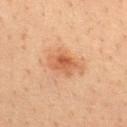The tile uses cross-polarized illumination. A male patient in their mid-30s. Located on the upper back. The recorded lesion diameter is about 5 mm. Cropped from a whole-body photographic skin survey; the tile spans about 15 mm. Automated image analysis of the tile measured an average lesion color of about L≈56 a*≈24 b*≈36 (CIELAB), about 10 CIELAB-L* units darker than the surrounding skin, and a lesion-to-skin contrast of about 7 (normalized; higher = more distinct).